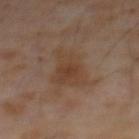Imaged during a routine full-body skin examination; the lesion was not biopsied and no histopathology is available. A close-up tile cropped from a whole-body skin photograph, about 15 mm across. The subject is a male aged 63–67. The lesion's longest dimension is about 6.5 mm. An algorithmic analysis of the crop reported a nevus-likeness score of about 0/100. Imaged with cross-polarized lighting.